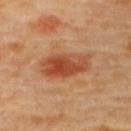biopsy status = total-body-photography surveillance lesion; no biopsy
body site = the upper back
lesion size = about 6 mm
imaging modality = ~15 mm crop, total-body skin-cancer survey
TBP lesion metrics = an area of roughly 14 mm² and a shape eccentricity near 0.9; internal color variation of about 7 on a 0–10 scale and a peripheral color-asymmetry measure near 2; a classifier nevus-likeness of about 100/100 and a lesion-detection confidence of about 100/100
subject = female, in their 60s